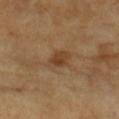{"biopsy_status": "not biopsied; imaged during a skin examination", "automated_metrics": {"color_variation_0_10": 4.5, "peripheral_color_asymmetry": 1.5}, "lesion_size": {"long_diameter_mm_approx": 3.0}, "site": "left forearm", "patient": {"sex": "female", "age_approx": 70}, "lighting": "cross-polarized", "image": {"source": "total-body photography crop", "field_of_view_mm": 15}}